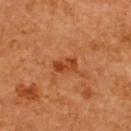Impression: Part of a total-body skin-imaging series; this lesion was reviewed on a skin check and was not flagged for biopsy. Image and clinical context: A close-up tile cropped from a whole-body skin photograph, about 15 mm across. The total-body-photography lesion software estimated an eccentricity of roughly 0.85 and a shape-asymmetry score of about 0.25 (0 = symmetric). And it measured a lesion color around L≈44 a*≈31 b*≈40 in CIELAB, about 10 CIELAB-L* units darker than the surrounding skin, and a lesion-to-skin contrast of about 7.5 (normalized; higher = more distinct). It also reported a border-irregularity index near 3/10, a color-variation rating of about 4/10, and radial color variation of about 1.5. It also reported a classifier nevus-likeness of about 45/100 and lesion-presence confidence of about 100/100. A female subject aged around 65. The lesion is located on the upper back. The tile uses cross-polarized illumination.